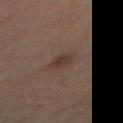Part of a total-body skin-imaging series; this lesion was reviewed on a skin check and was not flagged for biopsy.
A region of skin cropped from a whole-body photographic capture, roughly 15 mm wide.
The subject is a male roughly 65 years of age.
Automated image analysis of the tile measured a mean CIELAB color near L≈33 a*≈14 b*≈22, roughly 7 lightness units darker than nearby skin, and a normalized lesion–skin contrast near 7.
From the left thigh.